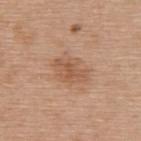Located on the upper back.
The lesion's longest dimension is about 3.5 mm.
This is a white-light tile.
An algorithmic analysis of the crop reported a mean CIELAB color near L≈55 a*≈21 b*≈33, roughly 8 lightness units darker than nearby skin, and a normalized border contrast of about 6. And it measured a within-lesion color-variation index near 3/10 and peripheral color asymmetry of about 1. And it measured a classifier nevus-likeness of about 5/100.
A region of skin cropped from a whole-body photographic capture, roughly 15 mm wide.
A male subject aged 68 to 72.